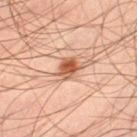follow-up: imaged on a skin check; not biopsied
automated lesion analysis: a mean CIELAB color near L≈53 a*≈25 b*≈34, a lesion–skin lightness drop of about 14, and a lesion-to-skin contrast of about 10 (normalized; higher = more distinct); border irregularity of about 2.5 on a 0–10 scale, a color-variation rating of about 6/10, and a peripheral color-asymmetry measure near 2
site: the left thigh
lighting: cross-polarized
acquisition: ~15 mm tile from a whole-body skin photo
patient: male, approximately 45 years of age
lesion diameter: ~2.5 mm (longest diameter)